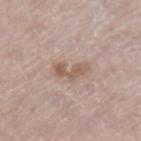biopsy status: imaged on a skin check; not biopsied | imaging modality: 15 mm crop, total-body photography | tile lighting: white-light | anatomic site: the leg | subject: male, aged around 65 | diameter: ~4 mm (longest diameter).